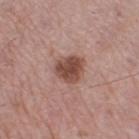Clinical impression: Captured during whole-body skin photography for melanoma surveillance; the lesion was not biopsied. Acquisition and patient details: A region of skin cropped from a whole-body photographic capture, roughly 15 mm wide. From the right thigh. A male subject, about 55 years old. Automated image analysis of the tile measured an area of roughly 9 mm², an outline eccentricity of about 0.3 (0 = round, 1 = elongated), and two-axis asymmetry of about 0.2. The software also gave an average lesion color of about L≈48 a*≈21 b*≈25 (CIELAB), about 13 CIELAB-L* units darker than the surrounding skin, and a lesion-to-skin contrast of about 9.5 (normalized; higher = more distinct). It also reported radial color variation of about 1. And it measured a nevus-likeness score of about 75/100.